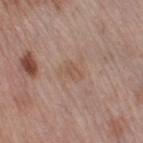Imaged during a routine full-body skin examination; the lesion was not biopsied and no histopathology is available. The tile uses white-light illumination. The subject is a female approximately 50 years of age. Approximately 3 mm at its widest. The lesion is located on the right thigh. This image is a 15 mm lesion crop taken from a total-body photograph.